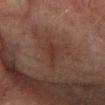| field | value |
|---|---|
| follow-up | total-body-photography surveillance lesion; no biopsy |
| image source | total-body-photography crop, ~15 mm field of view |
| diameter | about 4 mm |
| body site | the right forearm |
| subject | male, in their mid-70s |